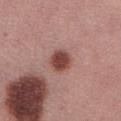<tbp_lesion>
<biopsy_status>not biopsied; imaged during a skin examination</biopsy_status>
<patient>
  <sex>female</sex>
  <age_approx>55</age_approx>
</patient>
<site>abdomen</site>
<image>
  <source>total-body photography crop</source>
  <field_of_view_mm>15</field_of_view_mm>
</image>
</tbp_lesion>